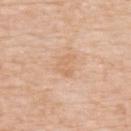follow-up: no biopsy performed (imaged during a skin exam) | patient: male, aged around 80 | image source: ~15 mm crop, total-body skin-cancer survey | anatomic site: the upper back | lesion size: about 3 mm | lighting: white-light illumination.